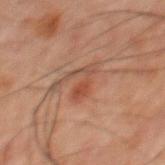Clinical impression: Part of a total-body skin-imaging series; this lesion was reviewed on a skin check and was not flagged for biopsy. Acquisition and patient details: The lesion is located on the back. The recorded lesion diameter is about 3.5 mm. Cropped from a whole-body photographic skin survey; the tile spans about 15 mm. A male patient about 60 years old. An algorithmic analysis of the crop reported an area of roughly 6 mm², a shape eccentricity near 0.8, and a shape-asymmetry score of about 0.55 (0 = symmetric). The analysis additionally found a lesion color around L≈37 a*≈19 b*≈24 in CIELAB and a lesion-to-skin contrast of about 5.5 (normalized; higher = more distinct). The analysis additionally found a nevus-likeness score of about 40/100 and lesion-presence confidence of about 100/100.